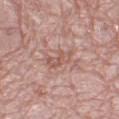Impression:
Imaged during a routine full-body skin examination; the lesion was not biopsied and no histopathology is available.
Context:
The lesion is on the leg. Cropped from a total-body skin-imaging series; the visible field is about 15 mm. A female patient in their mid- to late 50s. The tile uses white-light illumination.